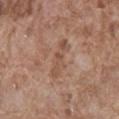| key | value |
|---|---|
| workup | no biopsy performed (imaged during a skin exam) |
| illumination | white-light illumination |
| size | ~5 mm (longest diameter) |
| image | ~15 mm crop, total-body skin-cancer survey |
| body site | the front of the torso |
| patient | male, about 75 years old |